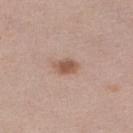Q: Is there a histopathology result?
A: total-body-photography surveillance lesion; no biopsy
Q: What is the imaging modality?
A: ~15 mm crop, total-body skin-cancer survey
Q: Lesion size?
A: ≈3 mm
Q: What lighting was used for the tile?
A: white-light
Q: Where on the body is the lesion?
A: the right lower leg
Q: Who is the patient?
A: female, about 40 years old